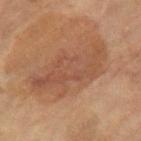- follow-up: catalogued during a skin exam; not biopsied
- image source: ~15 mm crop, total-body skin-cancer survey
- site: the right thigh
- lesion size: ≈9 mm
- subject: female, roughly 70 years of age
- image-analysis metrics: a footprint of about 26 mm², an outline eccentricity of about 0.9 (0 = round, 1 = elongated), and a symmetry-axis asymmetry near 0.4; a border-irregularity index near 7/10, internal color variation of about 4 on a 0–10 scale, and a peripheral color-asymmetry measure near 1.5; an automated nevus-likeness rating near 0 out of 100 and a lesion-detection confidence of about 100/100